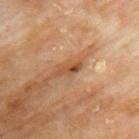Recorded during total-body skin imaging; not selected for excision or biopsy. A male subject aged approximately 70. A lesion tile, about 15 mm wide, cut from a 3D total-body photograph. The lesion is located on the back.